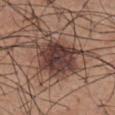Impression:
The lesion was tiled from a total-body skin photograph and was not biopsied.
Background:
A male patient aged around 55. The recorded lesion diameter is about 6.5 mm. The lesion is on the abdomen. A 15 mm crop from a total-body photograph taken for skin-cancer surveillance.